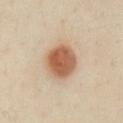Findings:
- biopsy status — imaged on a skin check; not biopsied
- subject — female, approximately 40 years of age
- lesion diameter — ~4.5 mm (longest diameter)
- TBP lesion metrics — a lesion color around L≈59 a*≈21 b*≈33 in CIELAB, a lesion–skin lightness drop of about 15, and a normalized border contrast of about 10
- body site — the chest
- image — 15 mm crop, total-body photography